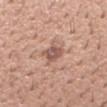workup: total-body-photography surveillance lesion; no biopsy | lighting: white-light illumination | patient: male, approximately 40 years of age | lesion diameter: about 3.5 mm | imaging modality: ~15 mm crop, total-body skin-cancer survey | TBP lesion metrics: an outline eccentricity of about 0.75 (0 = round, 1 = elongated); an average lesion color of about L≈55 a*≈21 b*≈25 (CIELAB), about 11 CIELAB-L* units darker than the surrounding skin, and a normalized lesion–skin contrast near 7.5; internal color variation of about 3 on a 0–10 scale and radial color variation of about 1 | location: the left forearm.